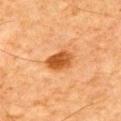{"biopsy_status": "not biopsied; imaged during a skin examination", "site": "chest", "lighting": "cross-polarized", "lesion_size": {"long_diameter_mm_approx": 3.5}, "automated_metrics": {"cielab_L": 46, "cielab_a": 25, "cielab_b": 40, "vs_skin_darker_L": 12.0, "vs_skin_contrast_norm": 10.0, "border_irregularity_0_10": 2.0, "color_variation_0_10": 3.5}, "patient": {"sex": "male", "age_approx": 70}, "image": {"source": "total-body photography crop", "field_of_view_mm": 15}}